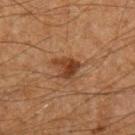follow-up = catalogued during a skin exam; not biopsied | patient = male, aged 48 to 52 | lighting = cross-polarized illumination | image = total-body-photography crop, ~15 mm field of view | location = the left upper arm | lesion size = about 3.5 mm.